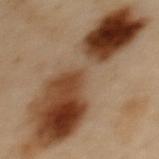Located on the upper back. Longest diameter approximately 13.5 mm. A female patient aged 58 to 62. A roughly 15 mm field-of-view crop from a total-body skin photograph.